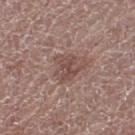* notes: catalogued during a skin exam; not biopsied
* subject: female, in their mid-60s
* size: ≈3.5 mm
* automated lesion analysis: a lesion area of about 7.5 mm², an eccentricity of roughly 0.45, and two-axis asymmetry of about 0.45; an average lesion color of about L≈48 a*≈19 b*≈22 (CIELAB) and a lesion–skin lightness drop of about 8; a within-lesion color-variation index near 3/10; a nevus-likeness score of about 0/100 and a detector confidence of about 100 out of 100 that the crop contains a lesion
* tile lighting: white-light illumination
* image: ~15 mm crop, total-body skin-cancer survey
* site: the left thigh